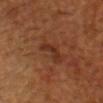Assessment: The lesion was photographed on a routine skin check and not biopsied; there is no pathology result. Clinical summary: Cropped from a total-body skin-imaging series; the visible field is about 15 mm. From the head or neck. Automated image analysis of the tile measured a lesion area of about 4 mm², a shape eccentricity near 0.9, and two-axis asymmetry of about 0.5. And it measured an average lesion color of about L≈29 a*≈21 b*≈28 (CIELAB). And it measured border irregularity of about 5.5 on a 0–10 scale, a within-lesion color-variation index near 0.5/10, and radial color variation of about 0. It also reported a nevus-likeness score of about 0/100. The recorded lesion diameter is about 3 mm. The subject is a male aged 53–57. Captured under cross-polarized illumination.